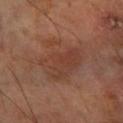| key | value |
|---|---|
| illumination | cross-polarized illumination |
| site | the arm |
| TBP lesion metrics | a border-irregularity rating of about 5/10, a within-lesion color-variation index near 4/10, and radial color variation of about 1.5; a detector confidence of about 100 out of 100 that the crop contains a lesion |
| imaging modality | ~15 mm crop, total-body skin-cancer survey |
| diameter | ~6 mm (longest diameter) |
| subject | male, approximately 70 years of age |